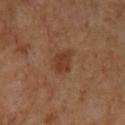Assessment: This lesion was catalogued during total-body skin photography and was not selected for biopsy. Background: The patient is a female aged approximately 50. Automated tile analysis of the lesion measured two-axis asymmetry of about 0.2. And it measured an average lesion color of about L≈39 a*≈23 b*≈31 (CIELAB), roughly 8 lightness units darker than nearby skin, and a normalized border contrast of about 7. A 15 mm close-up extracted from a 3D total-body photography capture. Longest diameter approximately 2.5 mm. The lesion is on the left lower leg.